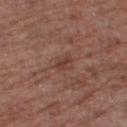Context: The lesion is on the right thigh. A 15 mm close-up tile from a total-body photography series done for melanoma screening. A male subject in their mid- to late 70s. Captured under white-light illumination. Approximately 2.5 mm at its widest. Automated image analysis of the tile measured a classifier nevus-likeness of about 0/100 and a lesion-detection confidence of about 95/100.